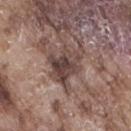Q: Was a biopsy performed?
A: no biopsy performed (imaged during a skin exam)
Q: How was the tile lit?
A: white-light
Q: Automated lesion metrics?
A: an average lesion color of about L≈41 a*≈16 b*≈19 (CIELAB) and a normalized lesion–skin contrast near 9.5; an automated nevus-likeness rating near 5 out of 100 and lesion-presence confidence of about 60/100
Q: Who is the patient?
A: male, roughly 75 years of age
Q: Where on the body is the lesion?
A: the right thigh
Q: What is the imaging modality?
A: total-body-photography crop, ~15 mm field of view
Q: Lesion size?
A: ~4 mm (longest diameter)